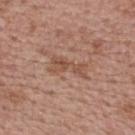notes — no biopsy performed (imaged during a skin exam)
image — total-body-photography crop, ~15 mm field of view
location — the upper back
lesion size — about 4.5 mm
subject — female, about 65 years old
automated metrics — an area of roughly 6.5 mm², an outline eccentricity of about 0.9 (0 = round, 1 = elongated), and a symmetry-axis asymmetry near 0.5; border irregularity of about 6.5 on a 0–10 scale, a color-variation rating of about 3.5/10, and peripheral color asymmetry of about 1.5; a detector confidence of about 100 out of 100 that the crop contains a lesion
illumination — white-light